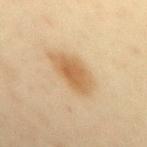Clinical impression:
Part of a total-body skin-imaging series; this lesion was reviewed on a skin check and was not flagged for biopsy.
Context:
The recorded lesion diameter is about 5 mm. A close-up tile cropped from a whole-body skin photograph, about 15 mm across. A female patient aged 43 to 47. From the back. Imaged with cross-polarized lighting.